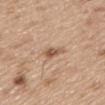biopsy status: no biopsy performed (imaged during a skin exam)
patient: male, approximately 70 years of age
site: the mid back
illumination: white-light illumination
image source: ~15 mm tile from a whole-body skin photo